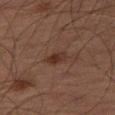| feature | finding |
|---|---|
| notes | imaged on a skin check; not biopsied |
| size | ≈3 mm |
| acquisition | 15 mm crop, total-body photography |
| site | the left thigh |
| subject | male, roughly 65 years of age |
| tile lighting | cross-polarized |